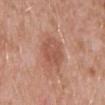About 4 mm across. A male subject, about 60 years old. The tile uses white-light illumination. This image is a 15 mm lesion crop taken from a total-body photograph. The lesion is on the abdomen.